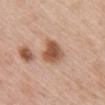{
  "biopsy_status": "not biopsied; imaged during a skin examination",
  "image": {
    "source": "total-body photography crop",
    "field_of_view_mm": 15
  },
  "lesion_size": {
    "long_diameter_mm_approx": 3.5
  },
  "site": "upper back",
  "lighting": "white-light",
  "patient": {
    "sex": "female",
    "age_approx": 50
  }
}